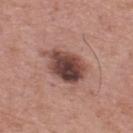Recorded during total-body skin imaging; not selected for excision or biopsy. A male patient, aged around 65. Approximately 5.5 mm at its widest. Cropped from a total-body skin-imaging series; the visible field is about 15 mm. The tile uses white-light illumination. The lesion is located on the upper back.